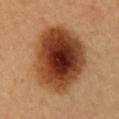Notes:
– workup: catalogued during a skin exam; not biopsied
– automated lesion analysis: a footprint of about 47 mm² and a shape-asymmetry score of about 0.15 (0 = symmetric)
– image source: ~15 mm tile from a whole-body skin photo
– location: the lower back
– lesion size: ~9 mm (longest diameter)
– tile lighting: cross-polarized illumination
– subject: female